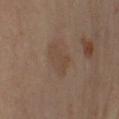workup: total-body-photography surveillance lesion; no biopsy
lighting: cross-polarized
lesion size: about 4 mm
location: the left arm
patient: female, in their mid-60s
imaging modality: ~15 mm crop, total-body skin-cancer survey